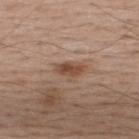- workup — total-body-photography surveillance lesion; no biopsy
- patient — male, approximately 40 years of age
- body site — the upper back
- lighting — white-light
- automated metrics — a footprint of about 4 mm², a shape eccentricity near 0.85, and a shape-asymmetry score of about 0.2 (0 = symmetric); about 11 CIELAB-L* units darker than the surrounding skin; a border-irregularity index near 2/10, a within-lesion color-variation index near 3/10, and radial color variation of about 1.5; an automated nevus-likeness rating near 95 out of 100 and a lesion-detection confidence of about 100/100
- diameter — ~3 mm (longest diameter)
- image source — total-body-photography crop, ~15 mm field of view